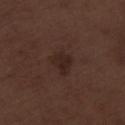Clinical impression:
No biopsy was performed on this lesion — it was imaged during a full skin examination and was not determined to be concerning.
Clinical summary:
The tile uses white-light illumination. This image is a 15 mm lesion crop taken from a total-body photograph. From the right lower leg. A male subject, in their 70s. The lesion's longest dimension is about 3 mm. The lesion-visualizer software estimated a nevus-likeness score of about 60/100.